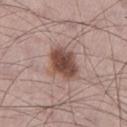From the left thigh. A lesion tile, about 15 mm wide, cut from a 3D total-body photograph. The patient is a male about 70 years old.